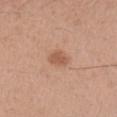Recorded during total-body skin imaging; not selected for excision or biopsy. About 2.5 mm across. The subject is a female aged 38 to 42. A roughly 15 mm field-of-view crop from a total-body skin photograph. From the left forearm. Imaged with white-light lighting. The lesion-visualizer software estimated a footprint of about 4.5 mm² and two-axis asymmetry of about 0.25. And it measured a mean CIELAB color near L≈56 a*≈22 b*≈31, a lesion–skin lightness drop of about 9, and a normalized border contrast of about 6.5. The software also gave a border-irregularity index near 2/10 and a color-variation rating of about 1/10.